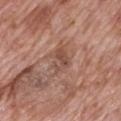The lesion was tiled from a total-body skin photograph and was not biopsied. The subject is a male aged around 70. A lesion tile, about 15 mm wide, cut from a 3D total-body photograph. The lesion is on the mid back.